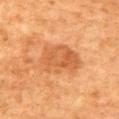Q: Is there a histopathology result?
A: total-body-photography surveillance lesion; no biopsy
Q: What did automated image analysis measure?
A: a footprint of about 16 mm², an eccentricity of roughly 0.75, and a shape-asymmetry score of about 0.2 (0 = symmetric); a lesion color around L≈46 a*≈22 b*≈35 in CIELAB, a lesion–skin lightness drop of about 8, and a normalized lesion–skin contrast near 6; a color-variation rating of about 3.5/10 and peripheral color asymmetry of about 1.5; a nevus-likeness score of about 20/100 and lesion-presence confidence of about 100/100
Q: How was the tile lit?
A: cross-polarized
Q: Lesion location?
A: the upper back
Q: Who is the patient?
A: male, aged 78–82
Q: How large is the lesion?
A: ≈5.5 mm
Q: How was this image acquired?
A: ~15 mm crop, total-body skin-cancer survey